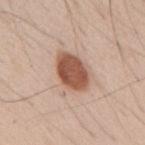Q: Was this lesion biopsied?
A: no biopsy performed (imaged during a skin exam)
Q: How was this image acquired?
A: 15 mm crop, total-body photography
Q: Lesion location?
A: the back
Q: What are the patient's age and sex?
A: male, aged 63 to 67
Q: How large is the lesion?
A: ≈5 mm
Q: What did automated image analysis measure?
A: a footprint of about 13 mm²; a mean CIELAB color near L≈54 a*≈22 b*≈29, about 16 CIELAB-L* units darker than the surrounding skin, and a lesion-to-skin contrast of about 10.5 (normalized; higher = more distinct); border irregularity of about 2 on a 0–10 scale, a within-lesion color-variation index near 3.5/10, and radial color variation of about 1; a nevus-likeness score of about 95/100 and a lesion-detection confidence of about 100/100